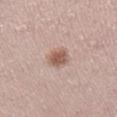{"lighting": "white-light", "site": "leg", "patient": {"sex": "female", "age_approx": 40}, "lesion_size": {"long_diameter_mm_approx": 2.5}, "image": {"source": "total-body photography crop", "field_of_view_mm": 15}}